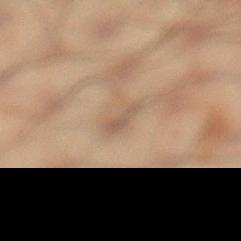{"biopsy_status": "not biopsied; imaged during a skin examination", "lesion_size": {"long_diameter_mm_approx": 2.5}, "automated_metrics": {"area_mm2_approx": 2.0, "eccentricity": 0.95, "shape_asymmetry": 0.4, "nevus_likeness_0_100": 0, "lesion_detection_confidence_0_100": 80}, "site": "left lower leg", "patient": {"sex": "male", "age_approx": 50}, "image": {"source": "total-body photography crop", "field_of_view_mm": 15}}